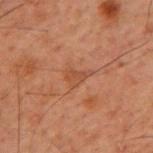No biopsy was performed on this lesion — it was imaged during a full skin examination and was not determined to be concerning. Cropped from a total-body skin-imaging series; the visible field is about 15 mm. A male subject in their 60s. Located on the upper back. The total-body-photography lesion software estimated a lesion color around L≈37 a*≈20 b*≈28 in CIELAB, about 5 CIELAB-L* units darker than the surrounding skin, and a normalized border contrast of about 5. It also reported a nevus-likeness score of about 0/100 and a lesion-detection confidence of about 100/100. The recorded lesion diameter is about 2.5 mm.